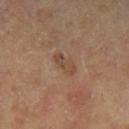biopsy status: imaged on a skin check; not biopsied
lighting: cross-polarized illumination
image-analysis metrics: an area of roughly 4.5 mm², an outline eccentricity of about 0.9 (0 = round, 1 = elongated), and a symmetry-axis asymmetry near 0.3; a normalized lesion–skin contrast near 5.5; border irregularity of about 3 on a 0–10 scale and a within-lesion color-variation index near 4.5/10
subject: male, approximately 65 years of age
location: the left lower leg
acquisition: total-body-photography crop, ~15 mm field of view
diameter: ~3 mm (longest diameter)